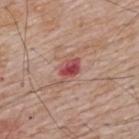Recorded during total-body skin imaging; not selected for excision or biopsy. A roughly 15 mm field-of-view crop from a total-body skin photograph. A male patient aged around 65. Located on the upper back. Longest diameter approximately 3 mm.